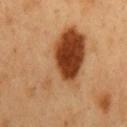Captured during whole-body skin photography for melanoma surveillance; the lesion was not biopsied.
Located on the mid back.
A 15 mm crop from a total-body photograph taken for skin-cancer surveillance.
About 10 mm across.
Automated image analysis of the tile measured about 14 CIELAB-L* units darker than the surrounding skin and a lesion-to-skin contrast of about 10 (normalized; higher = more distinct). The software also gave a within-lesion color-variation index near 10/10 and peripheral color asymmetry of about 5.
The tile uses cross-polarized illumination.
A male patient, aged around 50.